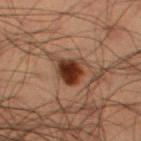Recorded during total-body skin imaging; not selected for excision or biopsy.
Imaged with cross-polarized lighting.
A male subject, aged around 50.
The lesion is on the left thigh.
This image is a 15 mm lesion crop taken from a total-body photograph.